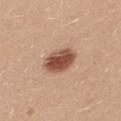follow-up: catalogued during a skin exam; not biopsied
acquisition: ~15 mm tile from a whole-body skin photo
patient: female, aged 23–27
diameter: about 4.5 mm
TBP lesion metrics: an area of roughly 9.5 mm² and a symmetry-axis asymmetry near 0.15; an automated nevus-likeness rating near 100 out of 100 and lesion-presence confidence of about 100/100
anatomic site: the upper back